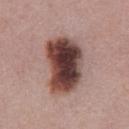This lesion was catalogued during total-body skin photography and was not selected for biopsy.
Approximately 6.5 mm at its widest.
A lesion tile, about 15 mm wide, cut from a 3D total-body photograph.
The lesion is on the abdomen.
A male subject aged approximately 60.